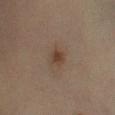Part of a total-body skin-imaging series; this lesion was reviewed on a skin check and was not flagged for biopsy.
The lesion is on the left lower leg.
The patient is a female aged 68 to 72.
A 15 mm close-up tile from a total-body photography series done for melanoma screening.